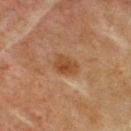Captured during whole-body skin photography for melanoma surveillance; the lesion was not biopsied. Approximately 3 mm at its widest. Imaged with cross-polarized lighting. Located on the front of the torso. A male subject, in their mid-70s. The lesion-visualizer software estimated about 7 CIELAB-L* units darker than the surrounding skin and a normalized border contrast of about 7.5. The software also gave a detector confidence of about 100 out of 100 that the crop contains a lesion. A 15 mm close-up tile from a total-body photography series done for melanoma screening.